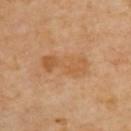follow-up: total-body-photography surveillance lesion; no biopsy
automated metrics: a lesion area of about 10 mm², an outline eccentricity of about 0.95 (0 = round, 1 = elongated), and a symmetry-axis asymmetry near 0.3; an average lesion color of about L≈54 a*≈21 b*≈37 (CIELAB) and about 7 CIELAB-L* units darker than the surrounding skin; a border-irregularity index near 5/10, internal color variation of about 3.5 on a 0–10 scale, and peripheral color asymmetry of about 1
lesion diameter: ~5.5 mm (longest diameter)
lighting: cross-polarized
site: the back
subject: male, roughly 70 years of age
imaging modality: total-body-photography crop, ~15 mm field of view